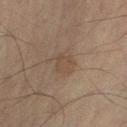Clinical summary: The total-body-photography lesion software estimated a lesion area of about 5.5 mm² and an outline eccentricity of about 0.65 (0 = round, 1 = elongated). The software also gave a mean CIELAB color near L≈37 a*≈12 b*≈22 and a normalized lesion–skin contrast near 4.5. This is a cross-polarized tile. The recorded lesion diameter is about 3 mm. A 15 mm close-up tile from a total-body photography series done for melanoma screening. On the right upper arm. A male patient aged approximately 70.